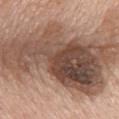Notes:
* notes — imaged on a skin check; not biopsied
* subject — female, approximately 60 years of age
* acquisition — total-body-photography crop, ~15 mm field of view
* illumination — white-light
* site — the mid back